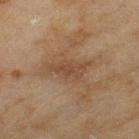A female subject aged 58–62.
The total-body-photography lesion software estimated a lesion area of about 7 mm². And it measured an average lesion color of about L≈42 a*≈17 b*≈29 (CIELAB), about 7 CIELAB-L* units darker than the surrounding skin, and a normalized lesion–skin contrast near 6. And it measured a border-irregularity rating of about 5.5/10, a color-variation rating of about 2/10, and peripheral color asymmetry of about 0.5. The software also gave a classifier nevus-likeness of about 0/100 and a lesion-detection confidence of about 95/100.
The lesion is on the left thigh.
This image is a 15 mm lesion crop taken from a total-body photograph.
Imaged with cross-polarized lighting.